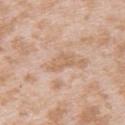A 15 mm close-up extracted from a 3D total-body photography capture.
From the upper back.
The subject is a female in their mid-20s.
This is a white-light tile.